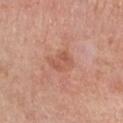Recorded during total-body skin imaging; not selected for excision or biopsy.
The lesion-visualizer software estimated a lesion–skin lightness drop of about 7 and a normalized lesion–skin contrast near 5. It also reported a border-irregularity index near 3/10, internal color variation of about 3 on a 0–10 scale, and peripheral color asymmetry of about 1.
The lesion is located on the right forearm.
This image is a 15 mm lesion crop taken from a total-body photograph.
A female subject, aged 63–67.
This is a white-light tile.